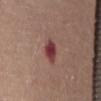Q: Was this lesion biopsied?
A: no biopsy performed (imaged during a skin exam)
Q: What is the imaging modality?
A: ~15 mm crop, total-body skin-cancer survey
Q: Lesion location?
A: the abdomen
Q: Who is the patient?
A: female, in their mid- to late 60s
Q: Automated lesion metrics?
A: a mean CIELAB color near L≈39 a*≈26 b*≈20